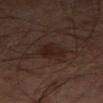biopsy status: no biopsy performed (imaged during a skin exam)
body site: the leg
imaging modality: total-body-photography crop, ~15 mm field of view
lesion size: ≈3.5 mm
patient: male, aged 58 to 62
illumination: cross-polarized illumination